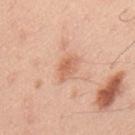No biopsy was performed on this lesion — it was imaged during a full skin examination and was not determined to be concerning. This image is a 15 mm lesion crop taken from a total-body photograph. From the mid back. Captured under white-light illumination. The patient is a male aged around 45. Automated image analysis of the tile measured an area of roughly 4 mm², an eccentricity of roughly 0.8, and a shape-asymmetry score of about 0.3 (0 = symmetric). It also reported an average lesion color of about L≈63 a*≈25 b*≈33 (CIELAB) and a normalized lesion–skin contrast near 6.5. It also reported a border-irregularity index near 3/10, a within-lesion color-variation index near 2.5/10, and a peripheral color-asymmetry measure near 0.5. The software also gave a classifier nevus-likeness of about 30/100. The lesion's longest dimension is about 3 mm.